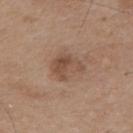biopsy status — catalogued during a skin exam; not biopsied
site — the back
subject — male, in their mid- to late 70s
image-analysis metrics — a lesion area of about 8.5 mm², a shape eccentricity near 0.65, and a shape-asymmetry score of about 0.35 (0 = symmetric); a border-irregularity rating of about 4/10, a color-variation rating of about 4.5/10, and a peripheral color-asymmetry measure near 1.5; a detector confidence of about 100 out of 100 that the crop contains a lesion
tile lighting — white-light illumination
size — ~3.5 mm (longest diameter)
imaging modality — ~15 mm crop, total-body skin-cancer survey